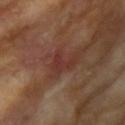Captured during whole-body skin photography for melanoma surveillance; the lesion was not biopsied. A 15 mm close-up tile from a total-body photography series done for melanoma screening. The lesion is located on the left upper arm. Imaged with cross-polarized lighting. The lesion's longest dimension is about 3 mm. A female subject approximately 75 years of age. Automated tile analysis of the lesion measured a color-variation rating of about 1/10 and radial color variation of about 0.5. The analysis additionally found a classifier nevus-likeness of about 0/100 and a detector confidence of about 95 out of 100 that the crop contains a lesion.